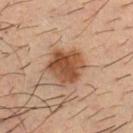• follow-up — catalogued during a skin exam; not biopsied
• location — the chest
• patient — male, in their mid- to late 30s
• lesion size — about 4.5 mm
• illumination — cross-polarized
• acquisition — ~15 mm crop, total-body skin-cancer survey
• automated lesion analysis — two-axis asymmetry of about 0.2; a border-irregularity rating of about 2/10, a color-variation rating of about 4.5/10, and peripheral color asymmetry of about 1.5